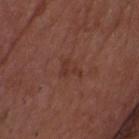notes: total-body-photography surveillance lesion; no biopsy | site: the head or neck | imaging modality: 15 mm crop, total-body photography | size: ≈2.5 mm | illumination: white-light | subject: male, about 50 years old.